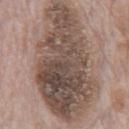Assessment: Imaged during a routine full-body skin examination; the lesion was not biopsied and no histopathology is available. Image and clinical context: A male subject aged around 70. Located on the front of the torso. The lesion-visualizer software estimated a footprint of about 75 mm², an eccentricity of roughly 0.9, and two-axis asymmetry of about 0.25. And it measured a lesion color around L≈49 a*≈14 b*≈23 in CIELAB and a lesion–skin lightness drop of about 14. A 15 mm crop from a total-body photograph taken for skin-cancer surveillance. Captured under white-light illumination. The recorded lesion diameter is about 15 mm.